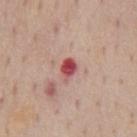The lesion was photographed on a routine skin check and not biopsied; there is no pathology result.
A roughly 15 mm field-of-view crop from a total-body skin photograph.
Longest diameter approximately 2.5 mm.
A male subject about 60 years old.
Located on the chest.
The lesion-visualizer software estimated a footprint of about 4 mm² and a symmetry-axis asymmetry near 0.3. The analysis additionally found a border-irregularity rating of about 2.5/10, internal color variation of about 5 on a 0–10 scale, and peripheral color asymmetry of about 1.5.
Captured under white-light illumination.